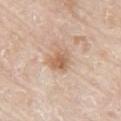- notes — catalogued during a skin exam; not biopsied